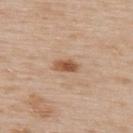patient: male, aged around 55 | automated lesion analysis: a footprint of about 4 mm², an outline eccentricity of about 0.85 (0 = round, 1 = elongated), and a symmetry-axis asymmetry near 0.25; a lesion color around L≈54 a*≈21 b*≈34 in CIELAB, about 13 CIELAB-L* units darker than the surrounding skin, and a normalized border contrast of about 9; a border-irregularity index near 2.5/10 and radial color variation of about 1 | site: the upper back | image: 15 mm crop, total-body photography.